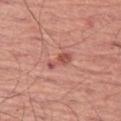Imaged during a routine full-body skin examination; the lesion was not biopsied and no histopathology is available.
The lesion's longest dimension is about 3.5 mm.
The patient is a male aged 63 to 67.
Captured under white-light illumination.
A 15 mm crop from a total-body photograph taken for skin-cancer surveillance.
Located on the left thigh.
An algorithmic analysis of the crop reported an area of roughly 3.5 mm². The analysis additionally found internal color variation of about 1.5 on a 0–10 scale and peripheral color asymmetry of about 0.5. The analysis additionally found an automated nevus-likeness rating near 10 out of 100 and lesion-presence confidence of about 100/100.